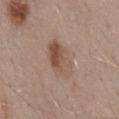{
  "biopsy_status": "not biopsied; imaged during a skin examination",
  "lighting": "white-light",
  "patient": {
    "sex": "male",
    "age_approx": 55
  },
  "image": {
    "source": "total-body photography crop",
    "field_of_view_mm": 15
  },
  "lesion_size": {
    "long_diameter_mm_approx": 5.0
  },
  "site": "back",
  "automated_metrics": {
    "eccentricity": 0.85,
    "shape_asymmetry": 0.15,
    "vs_skin_contrast_norm": 7.0,
    "border_irregularity_0_10": 1.5,
    "color_variation_0_10": 8.5,
    "peripheral_color_asymmetry": 3.5,
    "nevus_likeness_0_100": 60,
    "lesion_detection_confidence_0_100": 100
  }
}